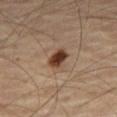biopsy status: imaged on a skin check; not biopsied
lesion size: ≈2.5 mm
subject: male, aged 63 to 67
site: the leg
imaging modality: ~15 mm tile from a whole-body skin photo
lighting: cross-polarized